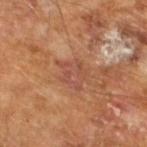This lesion was catalogued during total-body skin photography and was not selected for biopsy. Measured at roughly 4 mm in maximum diameter. A close-up tile cropped from a whole-body skin photograph, about 15 mm across. The patient is a male roughly 65 years of age.